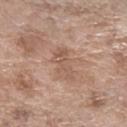Captured during whole-body skin photography for melanoma surveillance; the lesion was not biopsied. The patient is a female roughly 75 years of age. The lesion-visualizer software estimated a footprint of about 5.5 mm², a shape eccentricity near 0.85, and a shape-asymmetry score of about 0.4 (0 = symmetric). About 4 mm across. From the right forearm. A roughly 15 mm field-of-view crop from a total-body skin photograph.